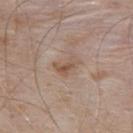Recorded during total-body skin imaging; not selected for excision or biopsy. A male patient roughly 55 years of age. A roughly 15 mm field-of-view crop from a total-body skin photograph. The total-body-photography lesion software estimated lesion-presence confidence of about 100/100. On the upper back. Longest diameter approximately 3 mm. This is a white-light tile.